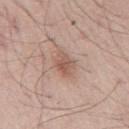Recorded during total-body skin imaging; not selected for excision or biopsy. The subject is a male in their mid-50s. The total-body-photography lesion software estimated a mean CIELAB color near L≈56 a*≈20 b*≈26, roughly 9 lightness units darker than nearby skin, and a normalized border contrast of about 6.5. This image is a 15 mm lesion crop taken from a total-body photograph. The lesion is located on the abdomen. About 3.5 mm across. The tile uses white-light illumination.